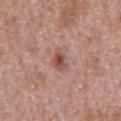Q: Is there a histopathology result?
A: total-body-photography surveillance lesion; no biopsy
Q: What are the patient's age and sex?
A: male, aged 63–67
Q: How was the tile lit?
A: white-light illumination
Q: Automated lesion metrics?
A: a lesion area of about 4.5 mm², an outline eccentricity of about 0.85 (0 = round, 1 = elongated), and two-axis asymmetry of about 0.3; a mean CIELAB color near L≈51 a*≈23 b*≈25 and a lesion-to-skin contrast of about 7.5 (normalized; higher = more distinct); a border-irregularity rating of about 3/10 and peripheral color asymmetry of about 2; a nevus-likeness score of about 5/100
Q: How large is the lesion?
A: ≈3 mm
Q: What is the anatomic site?
A: the front of the torso
Q: What kind of image is this?
A: ~15 mm crop, total-body skin-cancer survey